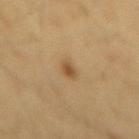Assessment: Part of a total-body skin-imaging series; this lesion was reviewed on a skin check and was not flagged for biopsy. Context: This is a cross-polarized tile. Located on the mid back. The lesion's longest dimension is about 2 mm. A male patient, in their mid- to late 60s. A 15 mm close-up tile from a total-body photography series done for melanoma screening.